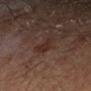Notes:
- notes: no biopsy performed (imaged during a skin exam)
- image source: ~15 mm tile from a whole-body skin photo
- automated metrics: a color-variation rating of about 1/10 and radial color variation of about 0.5
- anatomic site: the left forearm
- tile lighting: cross-polarized
- diameter: ≈2.5 mm
- patient: male, aged 63 to 67